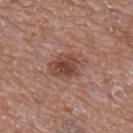Captured during whole-body skin photography for melanoma surveillance; the lesion was not biopsied. A male patient, in their 70s. From the upper back. Measured at roughly 4.5 mm in maximum diameter. Imaged with white-light lighting. An algorithmic analysis of the crop reported an area of roughly 10 mm², an outline eccentricity of about 0.7 (0 = round, 1 = elongated), and a shape-asymmetry score of about 0.15 (0 = symmetric). And it measured border irregularity of about 2.5 on a 0–10 scale and a within-lesion color-variation index near 4/10. The software also gave a classifier nevus-likeness of about 65/100 and a lesion-detection confidence of about 100/100. Cropped from a whole-body photographic skin survey; the tile spans about 15 mm.